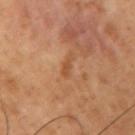Case summary:
- workup · no biopsy performed (imaged during a skin exam)
- diameter · ~3 mm (longest diameter)
- image · total-body-photography crop, ~15 mm field of view
- patient · male, about 50 years old
- location · the right upper arm
- illumination · cross-polarized illumination